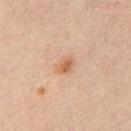Assessment:
The lesion was photographed on a routine skin check and not biopsied; there is no pathology result.
Acquisition and patient details:
A male subject, roughly 30 years of age. Approximately 2.5 mm at its widest. A 15 mm close-up extracted from a 3D total-body photography capture. Automated tile analysis of the lesion measured an area of roughly 4 mm² and two-axis asymmetry of about 0.25. The analysis additionally found an automated nevus-likeness rating near 80 out of 100. The tile uses cross-polarized illumination. On the chest.